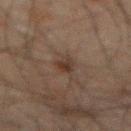The lesion was photographed on a routine skin check and not biopsied; there is no pathology result. The lesion is on the abdomen. The patient is a male roughly 60 years of age. A roughly 15 mm field-of-view crop from a total-body skin photograph.